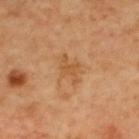The lesion was photographed on a routine skin check and not biopsied; there is no pathology result. Longest diameter approximately 3.5 mm. The lesion-visualizer software estimated an average lesion color of about L≈52 a*≈21 b*≈39 (CIELAB), about 7 CIELAB-L* units darker than the surrounding skin, and a normalized lesion–skin contrast near 6. The software also gave border irregularity of about 5.5 on a 0–10 scale and peripheral color asymmetry of about 0.5. The analysis additionally found a nevus-likeness score of about 0/100 and a lesion-detection confidence of about 100/100. Located on the upper back. Imaged with cross-polarized lighting. A male patient, about 60 years old. Cropped from a total-body skin-imaging series; the visible field is about 15 mm.